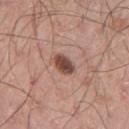Q: Was a biopsy performed?
A: total-body-photography surveillance lesion; no biopsy
Q: What is the imaging modality?
A: ~15 mm tile from a whole-body skin photo
Q: Who is the patient?
A: male, aged approximately 50
Q: Where on the body is the lesion?
A: the left thigh